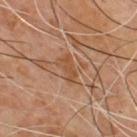Q: Was a biopsy performed?
A: no biopsy performed (imaged during a skin exam)
Q: How was the tile lit?
A: cross-polarized illumination
Q: What kind of image is this?
A: total-body-photography crop, ~15 mm field of view
Q: Where on the body is the lesion?
A: the chest
Q: What did automated image analysis measure?
A: a lesion color around L≈46 a*≈20 b*≈33 in CIELAB, a lesion–skin lightness drop of about 7, and a normalized lesion–skin contrast near 6.5; a border-irregularity rating of about 3.5/10, a color-variation rating of about 0.5/10, and a peripheral color-asymmetry measure near 0; an automated nevus-likeness rating near 0 out of 100 and a detector confidence of about 75 out of 100 that the crop contains a lesion
Q: Who is the patient?
A: male, approximately 55 years of age